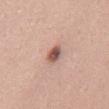Impression:
This lesion was catalogued during total-body skin photography and was not selected for biopsy.
Context:
The recorded lesion diameter is about 2.5 mm. The lesion is on the mid back. Cropped from a total-body skin-imaging series; the visible field is about 15 mm. The tile uses white-light illumination. The subject is a male aged around 55. Automated tile analysis of the lesion measured an average lesion color of about L≈55 a*≈21 b*≈25 (CIELAB), about 15 CIELAB-L* units darker than the surrounding skin, and a lesion-to-skin contrast of about 10 (normalized; higher = more distinct). And it measured border irregularity of about 2.5 on a 0–10 scale, internal color variation of about 5 on a 0–10 scale, and peripheral color asymmetry of about 1.5. The analysis additionally found a lesion-detection confidence of about 100/100.